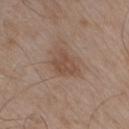biopsy status = catalogued during a skin exam; not biopsied
patient = male, roughly 55 years of age
illumination = white-light illumination
automated lesion analysis = a mean CIELAB color near L≈48 a*≈17 b*≈27, a lesion–skin lightness drop of about 8, and a normalized lesion–skin contrast near 6; a border-irregularity rating of about 3.5/10 and a color-variation rating of about 2/10; a detector confidence of about 100 out of 100 that the crop contains a lesion
anatomic site = the arm
image = total-body-photography crop, ~15 mm field of view